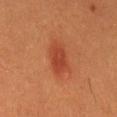  biopsy_status: not biopsied; imaged during a skin examination
  image:
    source: total-body photography crop
    field_of_view_mm: 15
  patient:
    sex: male
    age_approx: 60
  lighting: cross-polarized
  lesion_size:
    long_diameter_mm_approx: 4.5
  site: mid back
  automated_metrics:
    cielab_L: 42
    cielab_a: 30
    cielab_b: 34
    vs_skin_darker_L: 8.0
    vs_skin_contrast_norm: 6.5
    border_irregularity_0_10: 2.0
    color_variation_0_10: 2.5
    peripheral_color_asymmetry: 1.0
    nevus_likeness_0_100: 100
    lesion_detection_confidence_0_100: 100